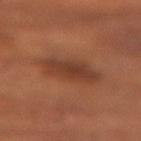Assessment:
The lesion was photographed on a routine skin check and not biopsied; there is no pathology result.
Acquisition and patient details:
A 15 mm close-up tile from a total-body photography series done for melanoma screening. From the left forearm. The lesion-visualizer software estimated an area of roughly 12 mm², an eccentricity of roughly 0.8, and a shape-asymmetry score of about 0.2 (0 = symmetric). The analysis additionally found an average lesion color of about L≈37 a*≈22 b*≈29 (CIELAB), about 8 CIELAB-L* units darker than the surrounding skin, and a lesion-to-skin contrast of about 7 (normalized; higher = more distinct). It also reported a border-irregularity index near 2/10 and a peripheral color-asymmetry measure near 1. Imaged with cross-polarized lighting. A male subject in their mid- to late 50s. The lesion's longest dimension is about 5 mm.